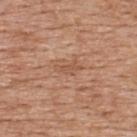This lesion was catalogued during total-body skin photography and was not selected for biopsy. A male patient, approximately 60 years of age. A lesion tile, about 15 mm wide, cut from a 3D total-body photograph. About 3 mm across. The lesion is located on the upper back.